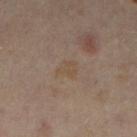Q: Was a biopsy performed?
A: no biopsy performed (imaged during a skin exam)
Q: What is the anatomic site?
A: the left lower leg
Q: What is the imaging modality?
A: 15 mm crop, total-body photography
Q: What lighting was used for the tile?
A: cross-polarized illumination
Q: What are the patient's age and sex?
A: female, aged 33 to 37
Q: What did automated image analysis measure?
A: an area of roughly 4 mm², an outline eccentricity of about 0.75 (0 = round, 1 = elongated), and a shape-asymmetry score of about 0.35 (0 = symmetric); a mean CIELAB color near L≈45 a*≈13 b*≈27, roughly 4 lightness units darker than nearby skin, and a normalized lesion–skin contrast near 5
Q: Lesion size?
A: about 2.5 mm